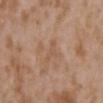This lesion was catalogued during total-body skin photography and was not selected for biopsy. Imaged with white-light lighting. The lesion is on the upper back. A roughly 15 mm field-of-view crop from a total-body skin photograph. A female subject approximately 35 years of age. Automated image analysis of the tile measured a footprint of about 3 mm², an eccentricity of roughly 0.9, and a shape-asymmetry score of about 0.7 (0 = symmetric). The analysis additionally found an average lesion color of about L≈54 a*≈18 b*≈31 (CIELAB), a lesion–skin lightness drop of about 6, and a normalized border contrast of about 4.5. The analysis additionally found lesion-presence confidence of about 100/100.